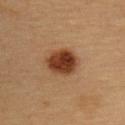Q: What lighting was used for the tile?
A: cross-polarized illumination
Q: How large is the lesion?
A: ≈4 mm
Q: What is the imaging modality?
A: ~15 mm crop, total-body skin-cancer survey
Q: Where on the body is the lesion?
A: the upper back
Q: Patient demographics?
A: female, about 30 years old